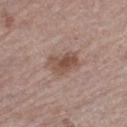acquisition: total-body-photography crop, ~15 mm field of view; location: the left thigh; subject: male, roughly 75 years of age.